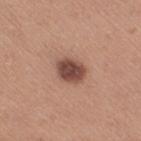Recorded during total-body skin imaging; not selected for excision or biopsy. From the right thigh. The total-body-photography lesion software estimated a lesion area of about 8 mm² and a symmetry-axis asymmetry near 0.2. Captured under white-light illumination. A 15 mm close-up extracted from a 3D total-body photography capture. A female subject, aged around 55. Longest diameter approximately 3.5 mm.